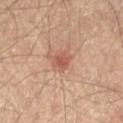* follow-up — no biopsy performed (imaged during a skin exam)
* anatomic site — the right thigh
* TBP lesion metrics — a footprint of about 4 mm², an eccentricity of roughly 0.6, and a shape-asymmetry score of about 0.25 (0 = symmetric)
* patient — male, aged around 65
* diameter — ≈2.5 mm
* image source — ~15 mm crop, total-body skin-cancer survey